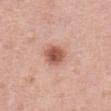workup: imaged on a skin check; not biopsied
image: total-body-photography crop, ~15 mm field of view
lighting: white-light illumination
subject: female, aged approximately 40
anatomic site: the front of the torso